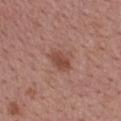Automated tile analysis of the lesion measured an area of roughly 7 mm² and a shape-asymmetry score of about 0.3 (0 = symmetric). And it measured about 9 CIELAB-L* units darker than the surrounding skin and a normalized lesion–skin contrast near 6.5. Captured under white-light illumination. About 3.5 mm across. The lesion is located on the back. A 15 mm crop from a total-body photograph taken for skin-cancer surveillance. A female patient in their 50s.